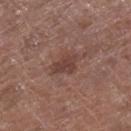- follow-up — catalogued during a skin exam; not biopsied
- acquisition — total-body-photography crop, ~15 mm field of view
- location — the leg
- subject — female, aged approximately 80
- automated metrics — a footprint of about 5 mm² and an eccentricity of roughly 0.7; an average lesion color of about L≈41 a*≈20 b*≈23 (CIELAB), about 8 CIELAB-L* units darker than the surrounding skin, and a normalized border contrast of about 7
- diameter — about 3.5 mm
- lighting — white-light illumination